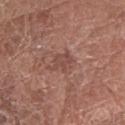  biopsy_status: not biopsied; imaged during a skin examination
  lighting: white-light
  automated_metrics:
    eccentricity: 0.55
    shape_asymmetry: 0.3
    border_irregularity_0_10: 3.5
    color_variation_0_10: 1.5
    peripheral_color_asymmetry: 0.5
    lesion_detection_confidence_0_100: 100
  lesion_size:
    long_diameter_mm_approx: 3.0
  image:
    source: total-body photography crop
    field_of_view_mm: 15
  patient:
    sex: female
    age_approx: 80
  site: right forearm